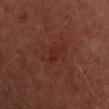Notes:
- biopsy status · catalogued during a skin exam; not biopsied